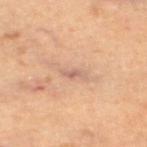Notes:
– notes — catalogued during a skin exam; not biopsied
– anatomic site — the left thigh
– automated metrics — a classifier nevus-likeness of about 0/100
– patient — male, aged approximately 60
– imaging modality — ~15 mm tile from a whole-body skin photo